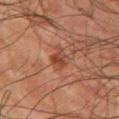Notes:
• workup: total-body-photography surveillance lesion; no biopsy
• body site: the left upper arm
• subject: male, in their mid-70s
• lesion diameter: about 3.5 mm
• acquisition: 15 mm crop, total-body photography
• tile lighting: cross-polarized illumination